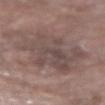Q: Was this lesion biopsied?
A: imaged on a skin check; not biopsied
Q: What kind of image is this?
A: total-body-photography crop, ~15 mm field of view
Q: Automated lesion metrics?
A: an eccentricity of roughly 0.65 and two-axis asymmetry of about 0.3
Q: What is the anatomic site?
A: the right forearm
Q: Patient demographics?
A: female, approximately 70 years of age
Q: How large is the lesion?
A: ≈8 mm